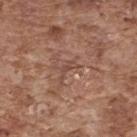Assessment:
Part of a total-body skin-imaging series; this lesion was reviewed on a skin check and was not flagged for biopsy.
Clinical summary:
A 15 mm crop from a total-body photograph taken for skin-cancer surveillance. The lesion is on the back. A male patient about 75 years old.